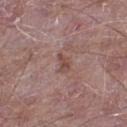Q: Was this lesion biopsied?
A: no biopsy performed (imaged during a skin exam)
Q: What is the lesion's diameter?
A: about 2.5 mm
Q: What is the anatomic site?
A: the leg
Q: Who is the patient?
A: male, approximately 65 years of age
Q: How was this image acquired?
A: total-body-photography crop, ~15 mm field of view
Q: What did automated image analysis measure?
A: an average lesion color of about L≈47 a*≈20 b*≈22 (CIELAB), a lesion–skin lightness drop of about 8, and a lesion-to-skin contrast of about 6.5 (normalized; higher = more distinct); a within-lesion color-variation index near 1/10